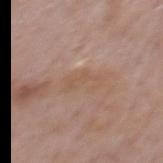body site: the back; patient: male, in their mid-70s; image source: total-body-photography crop, ~15 mm field of view.